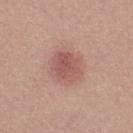{"biopsy_status": "not biopsied; imaged during a skin examination", "site": "right thigh", "patient": {"sex": "female", "age_approx": 20}, "automated_metrics": {"area_mm2_approx": 10.0, "cielab_L": 54, "cielab_a": 24, "cielab_b": 24, "vs_skin_darker_L": 9.0, "vs_skin_contrast_norm": 6.5}, "lesion_size": {"long_diameter_mm_approx": 3.5}, "lighting": "white-light", "image": {"source": "total-body photography crop", "field_of_view_mm": 15}}